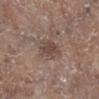Impression: No biopsy was performed on this lesion — it was imaged during a full skin examination and was not determined to be concerning.